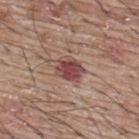Notes:
- biopsy status · no biopsy performed (imaged during a skin exam)
- subject · male, roughly 65 years of age
- lesion size · ~3.5 mm (longest diameter)
- imaging modality · ~15 mm crop, total-body skin-cancer survey
- TBP lesion metrics · a border-irregularity rating of about 1.5/10 and peripheral color asymmetry of about 1.5
- location · the back